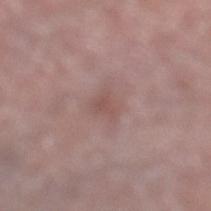biopsy status: imaged on a skin check; not biopsied | TBP lesion metrics: an area of roughly 4 mm², an outline eccentricity of about 0.55 (0 = round, 1 = elongated), and a symmetry-axis asymmetry near 0.3 | size: about 2.5 mm | patient: male, aged 73–77 | tile lighting: white-light illumination | anatomic site: the leg | imaging modality: 15 mm crop, total-body photography.